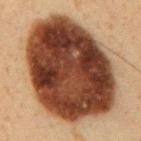Findings:
• notes — no biopsy performed (imaged during a skin exam)
• image-analysis metrics — a lesion color around L≈34 a*≈19 b*≈27 in CIELAB, about 22 CIELAB-L* units darker than the surrounding skin, and a normalized border contrast of about 17.5; a border-irregularity index near 1.5/10 and a within-lesion color-variation index near 8.5/10; a detector confidence of about 100 out of 100 that the crop contains a lesion
• acquisition — ~15 mm tile from a whole-body skin photo
• anatomic site — the mid back
• tile lighting — cross-polarized
• subject — male, aged approximately 60
• diameter — ≈12.5 mm